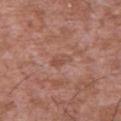Q: Was a biopsy performed?
A: imaged on a skin check; not biopsied
Q: What are the patient's age and sex?
A: male, aged around 45
Q: How was this image acquired?
A: 15 mm crop, total-body photography
Q: What is the anatomic site?
A: the chest
Q: How was the tile lit?
A: white-light illumination
Q: What did automated image analysis measure?
A: an average lesion color of about L≈50 a*≈22 b*≈27 (CIELAB) and a lesion–skin lightness drop of about 7; a border-irregularity rating of about 3/10, a color-variation rating of about 1.5/10, and radial color variation of about 0.5; an automated nevus-likeness rating near 0 out of 100
Q: How large is the lesion?
A: ≈2.5 mm